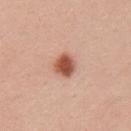workup = total-body-photography surveillance lesion; no biopsy | acquisition = ~15 mm tile from a whole-body skin photo | lesion size = ~2.5 mm (longest diameter) | tile lighting = white-light | subject = female, aged around 25 | body site = the left upper arm.